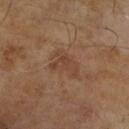The lesion was tiled from a total-body skin photograph and was not biopsied. A 15 mm crop from a total-body photograph taken for skin-cancer surveillance. An algorithmic analysis of the crop reported an outline eccentricity of about 0.8 (0 = round, 1 = elongated) and a symmetry-axis asymmetry near 0.35. The patient is a male in their mid- to late 60s. Measured at roughly 4 mm in maximum diameter.